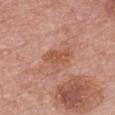Q: Is there a histopathology result?
A: catalogued during a skin exam; not biopsied
Q: What is the lesion's diameter?
A: ≈3.5 mm
Q: What kind of image is this?
A: ~15 mm crop, total-body skin-cancer survey
Q: Where on the body is the lesion?
A: the front of the torso
Q: What lighting was used for the tile?
A: white-light illumination
Q: Who is the patient?
A: female, roughly 60 years of age